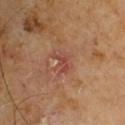workup: total-body-photography surveillance lesion; no biopsy | patient: male, about 60 years old | body site: the upper back | image-analysis metrics: a mean CIELAB color near L≈44 a*≈25 b*≈28, roughly 7 lightness units darker than nearby skin, and a lesion-to-skin contrast of about 5.5 (normalized; higher = more distinct); a border-irregularity index near 4.5/10 and radial color variation of about 0.5 | acquisition: ~15 mm crop, total-body skin-cancer survey.